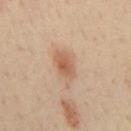The lesion was photographed on a routine skin check and not biopsied; there is no pathology result. A male subject, aged around 45. A region of skin cropped from a whole-body photographic capture, roughly 15 mm wide. The lesion is on the mid back.